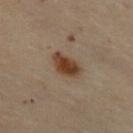This lesion was catalogued during total-body skin photography and was not selected for biopsy. A female patient in their 50s. A close-up tile cropped from a whole-body skin photograph, about 15 mm across. From the chest.